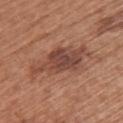{"biopsy_status": "not biopsied; imaged during a skin examination", "patient": {"sex": "female", "age_approx": 65}, "lighting": "white-light", "site": "back", "image": {"source": "total-body photography crop", "field_of_view_mm": 15}, "lesion_size": {"long_diameter_mm_approx": 7.5}}